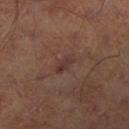Findings:
- follow-up: imaged on a skin check; not biopsied
- lesion size: ≈2.5 mm
- automated metrics: a lesion area of about 3 mm², an outline eccentricity of about 0.9 (0 = round, 1 = elongated), and a shape-asymmetry score of about 0.25 (0 = symmetric); a lesion–skin lightness drop of about 6 and a normalized lesion–skin contrast near 6.5
- imaging modality: total-body-photography crop, ~15 mm field of view
- site: the leg
- patient: male, roughly 65 years of age
- lighting: cross-polarized illumination